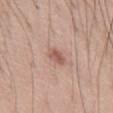Recorded during total-body skin imaging; not selected for excision or biopsy.
Imaged with white-light lighting.
The lesion is located on the abdomen.
Automated tile analysis of the lesion measured a classifier nevus-likeness of about 25/100 and a lesion-detection confidence of about 100/100.
Longest diameter approximately 2.5 mm.
Cropped from a whole-body photographic skin survey; the tile spans about 15 mm.
The subject is a male approximately 50 years of age.